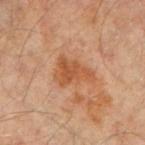The lesion was tiled from a total-body skin photograph and was not biopsied.
The recorded lesion diameter is about 4.5 mm.
Automated image analysis of the tile measured a symmetry-axis asymmetry near 0.45. The software also gave a detector confidence of about 100 out of 100 that the crop contains a lesion.
On the right upper arm.
Captured under cross-polarized illumination.
Cropped from a total-body skin-imaging series; the visible field is about 15 mm.
A male subject in their mid- to late 60s.